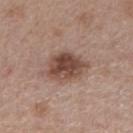Imaged during a routine full-body skin examination; the lesion was not biopsied and no histopathology is available.
This is a white-light tile.
Cropped from a whole-body photographic skin survey; the tile spans about 15 mm.
The patient is a male aged approximately 55.
The total-body-photography lesion software estimated a mean CIELAB color near L≈47 a*≈19 b*≈25, roughly 13 lightness units darker than nearby skin, and a normalized border contrast of about 9.5. It also reported a border-irregularity rating of about 2/10, a within-lesion color-variation index near 5.5/10, and a peripheral color-asymmetry measure near 2. The software also gave a nevus-likeness score of about 90/100 and a lesion-detection confidence of about 100/100.